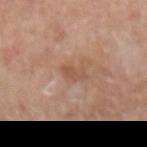Imaged during a routine full-body skin examination; the lesion was not biopsied and no histopathology is available. An algorithmic analysis of the crop reported a mean CIELAB color near L≈53 a*≈21 b*≈30 and a lesion-to-skin contrast of about 5.5 (normalized; higher = more distinct). The software also gave a border-irregularity rating of about 5/10 and peripheral color asymmetry of about 0. The tile uses white-light illumination. The recorded lesion diameter is about 3 mm. A male patient approximately 65 years of age. The lesion is located on the right forearm. Cropped from a whole-body photographic skin survey; the tile spans about 15 mm.